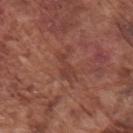notes = imaged on a skin check; not biopsied | image source = total-body-photography crop, ~15 mm field of view | site = the right upper arm | lesion diameter = about 4.5 mm | TBP lesion metrics = a classifier nevus-likeness of about 0/100 | patient = male, about 75 years old | tile lighting = white-light illumination.